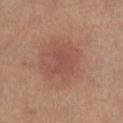Assessment:
Recorded during total-body skin imaging; not selected for excision or biopsy.
Clinical summary:
Longest diameter approximately 3 mm. The tile uses cross-polarized illumination. A close-up tile cropped from a whole-body skin photograph, about 15 mm across. A female patient about 30 years old. The lesion is located on the right lower leg.